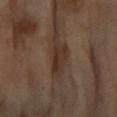Recorded during total-body skin imaging; not selected for excision or biopsy.
The lesion is located on the left forearm.
A female subject in their 70s.
Cropped from a whole-body photographic skin survey; the tile spans about 15 mm.